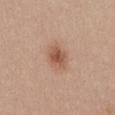About 3.5 mm across. The lesion is on the abdomen. Captured under white-light illumination. Automated tile analysis of the lesion measured a lesion color around L≈54 a*≈22 b*≈30 in CIELAB, roughly 11 lightness units darker than nearby skin, and a normalized border contrast of about 7.5. The analysis additionally found a border-irregularity index near 2/10. And it measured a nevus-likeness score of about 95/100 and a lesion-detection confidence of about 100/100. A female subject aged 18–22. Cropped from a whole-body photographic skin survey; the tile spans about 15 mm.